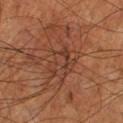Impression: Imaged during a routine full-body skin examination; the lesion was not biopsied and no histopathology is available. Clinical summary: A male subject in their mid- to late 60s. Cropped from a whole-body photographic skin survey; the tile spans about 15 mm. The lesion is on the left leg.